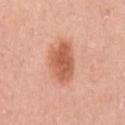This lesion was catalogued during total-body skin photography and was not selected for biopsy.
About 4.5 mm across.
The subject is a female roughly 40 years of age.
Cropped from a whole-body photographic skin survey; the tile spans about 15 mm.
Located on the left upper arm.
This is a white-light tile.
The lesion-visualizer software estimated a lesion area of about 13 mm², a shape eccentricity near 0.65, and a symmetry-axis asymmetry near 0.2.